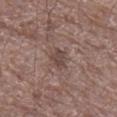Part of a total-body skin-imaging series; this lesion was reviewed on a skin check and was not flagged for biopsy. Captured under white-light illumination. A male patient, aged 68–72. An algorithmic analysis of the crop reported an outline eccentricity of about 0.75 (0 = round, 1 = elongated) and a symmetry-axis asymmetry near 0.2. The analysis additionally found a border-irregularity index near 2/10 and a peripheral color-asymmetry measure near 1. From the leg. The lesion's longest dimension is about 3 mm. This image is a 15 mm lesion crop taken from a total-body photograph.